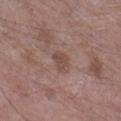Q: What did automated image analysis measure?
A: a lesion area of about 3.5 mm², an eccentricity of roughly 0.7, and a shape-asymmetry score of about 0.45 (0 = symmetric); a lesion color around L≈47 a*≈18 b*≈22 in CIELAB and about 8 CIELAB-L* units darker than the surrounding skin; internal color variation of about 1 on a 0–10 scale and a peripheral color-asymmetry measure near 0.5; a classifier nevus-likeness of about 0/100
Q: What kind of image is this?
A: ~15 mm tile from a whole-body skin photo
Q: Illumination type?
A: white-light
Q: Lesion location?
A: the right lower leg
Q: What are the patient's age and sex?
A: male, about 85 years old or older